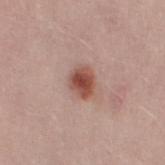Notes:
* notes: total-body-photography surveillance lesion; no biopsy
* tile lighting: white-light illumination
* location: the leg
* image: ~15 mm crop, total-body skin-cancer survey
* image-analysis metrics: a lesion area of about 6.5 mm²; a mean CIELAB color near L≈50 a*≈24 b*≈27, a lesion–skin lightness drop of about 14, and a lesion-to-skin contrast of about 10 (normalized; higher = more distinct)
* patient: female, aged 23–27